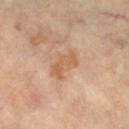Q: Was this lesion biopsied?
A: imaged on a skin check; not biopsied
Q: What kind of image is this?
A: total-body-photography crop, ~15 mm field of view
Q: Where on the body is the lesion?
A: the right lower leg
Q: What are the patient's age and sex?
A: female, aged approximately 60